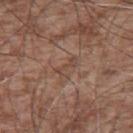The lesion was photographed on a routine skin check and not biopsied; there is no pathology result.
Approximately 3 mm at its widest.
The lesion is located on the upper back.
The tile uses white-light illumination.
This image is a 15 mm lesion crop taken from a total-body photograph.
A male subject, aged 53–57.
The total-body-photography lesion software estimated a footprint of about 3 mm², a shape eccentricity near 0.95, and a symmetry-axis asymmetry near 0.45. It also reported a border-irregularity index near 6.5/10 and internal color variation of about 0 on a 0–10 scale. The software also gave an automated nevus-likeness rating near 0 out of 100.